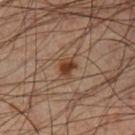Notes:
• follow-up — imaged on a skin check; not biopsied
• anatomic site — the left lower leg
• size — ≈2.5 mm
• image — total-body-photography crop, ~15 mm field of view
• lighting — cross-polarized
• subject — male, approximately 45 years of age